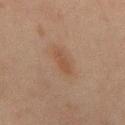Imaged during a routine full-body skin examination; the lesion was not biopsied and no histopathology is available. A male patient about 45 years old. A lesion tile, about 15 mm wide, cut from a 3D total-body photograph. Measured at roughly 3 mm in maximum diameter. The lesion is on the mid back. The tile uses cross-polarized illumination.